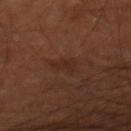Clinical impression: No biopsy was performed on this lesion — it was imaged during a full skin examination and was not determined to be concerning. Image and clinical context: A male subject aged 68 to 72. The lesion-visualizer software estimated an area of roughly 3.5 mm² and a shape-asymmetry score of about 0.4 (0 = symmetric). It also reported a lesion-to-skin contrast of about 5 (normalized; higher = more distinct). Located on the right thigh. Cropped from a whole-body photographic skin survey; the tile spans about 15 mm. This is a cross-polarized tile.